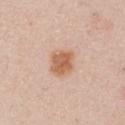Q: Was this lesion biopsied?
A: imaged on a skin check; not biopsied
Q: Where on the body is the lesion?
A: the chest
Q: Patient demographics?
A: male, in their 60s
Q: Illumination type?
A: white-light
Q: What is the imaging modality?
A: ~15 mm crop, total-body skin-cancer survey